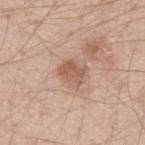The lesion was tiled from a total-body skin photograph and was not biopsied. The subject is a male in their 30s. A lesion tile, about 15 mm wide, cut from a 3D total-body photograph. The lesion is located on the left upper arm. Imaged with white-light lighting. Longest diameter approximately 3 mm. Automated tile analysis of the lesion measured an outline eccentricity of about 0.6 (0 = round, 1 = elongated) and a symmetry-axis asymmetry near 0.2. The analysis additionally found border irregularity of about 2.5 on a 0–10 scale, internal color variation of about 3.5 on a 0–10 scale, and radial color variation of about 1. The analysis additionally found a classifier nevus-likeness of about 25/100 and a detector confidence of about 100 out of 100 that the crop contains a lesion.